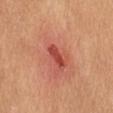Findings:
• workup · no biopsy performed (imaged during a skin exam)
• site · the lower back
• image · total-body-photography crop, ~15 mm field of view
• patient · female, aged approximately 60
• tile lighting · white-light illumination
• image-analysis metrics · a shape-asymmetry score of about 0.2 (0 = symmetric); a lesion color around L≈49 a*≈37 b*≈32 in CIELAB and about 11 CIELAB-L* units darker than the surrounding skin; a detector confidence of about 100 out of 100 that the crop contains a lesion
• lesion diameter · ≈3.5 mm